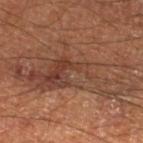No biopsy was performed on this lesion — it was imaged during a full skin examination and was not determined to be concerning. Located on the left lower leg. The recorded lesion diameter is about 14.5 mm. Captured under cross-polarized illumination. Cropped from a total-body skin-imaging series; the visible field is about 15 mm. The subject is a male aged 58 to 62.